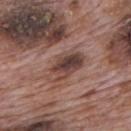lesion size = about 5 mm | illumination = white-light | acquisition = ~15 mm crop, total-body skin-cancer survey | body site = the mid back | patient = male, approximately 70 years of age.